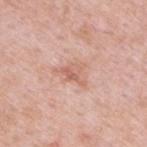biopsy status: imaged on a skin check; not biopsied
anatomic site: the upper back
patient: male, roughly 50 years of age
lighting: white-light
automated lesion analysis: a lesion area of about 3.5 mm² and an eccentricity of roughly 0.7; a border-irregularity rating of about 5/10, a color-variation rating of about 3.5/10, and a peripheral color-asymmetry measure near 1
imaging modality: ~15 mm crop, total-body skin-cancer survey
size: about 3 mm